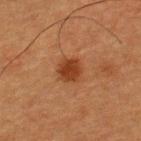biopsy status — imaged on a skin check; not biopsied
acquisition — total-body-photography crop, ~15 mm field of view
subject — male, aged 48 to 52
tile lighting — cross-polarized illumination
diameter — ≈2.5 mm
TBP lesion metrics — a lesion area of about 6 mm², an outline eccentricity of about 0.25 (0 = round, 1 = elongated), and a symmetry-axis asymmetry near 0.15; a classifier nevus-likeness of about 100/100 and a detector confidence of about 100 out of 100 that the crop contains a lesion
location — the upper back